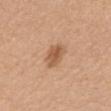Imaged during a routine full-body skin examination; the lesion was not biopsied and no histopathology is available. This is a white-light tile. Located on the mid back. A female patient, aged 53 to 57. Longest diameter approximately 3.5 mm. A lesion tile, about 15 mm wide, cut from a 3D total-body photograph.